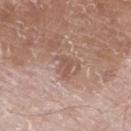| field | value |
|---|---|
| image source | ~15 mm crop, total-body skin-cancer survey |
| location | the leg |
| patient | male, aged 78 to 82 |